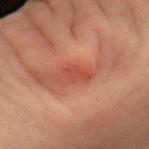{"image": {"source": "total-body photography crop", "field_of_view_mm": 15}, "lesion_size": {"long_diameter_mm_approx": 4.0}, "site": "left forearm", "lighting": "cross-polarized", "patient": {"sex": "female", "age_approx": 60}}